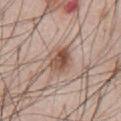follow-up: total-body-photography surveillance lesion; no biopsy
image source: 15 mm crop, total-body photography
patient: male, about 60 years old
image-analysis metrics: an area of roughly 6.5 mm², an outline eccentricity of about 0.75 (0 = round, 1 = elongated), and a shape-asymmetry score of about 0.25 (0 = symmetric); an average lesion color of about L≈51 a*≈19 b*≈27 (CIELAB), roughly 13 lightness units darker than nearby skin, and a normalized lesion–skin contrast near 9.5
illumination: white-light illumination
lesion size: ~3.5 mm (longest diameter)
anatomic site: the abdomen